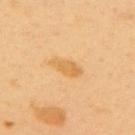Q: Was a biopsy performed?
A: imaged on a skin check; not biopsied
Q: Lesion location?
A: the upper back
Q: What are the patient's age and sex?
A: female, aged approximately 50
Q: What did automated image analysis measure?
A: a lesion color around L≈66 a*≈20 b*≈46 in CIELAB; a border-irregularity rating of about 3/10 and peripheral color asymmetry of about 0.5; a nevus-likeness score of about 55/100 and lesion-presence confidence of about 100/100
Q: What kind of image is this?
A: ~15 mm crop, total-body skin-cancer survey
Q: What is the lesion's diameter?
A: ≈4 mm
Q: How was the tile lit?
A: cross-polarized